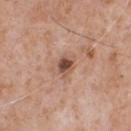Imaged during a routine full-body skin examination; the lesion was not biopsied and no histopathology is available. Longest diameter approximately 2.5 mm. Captured under white-light illumination. A region of skin cropped from a whole-body photographic capture, roughly 15 mm wide. On the front of the torso. A male subject aged 63 to 67.